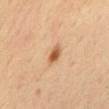A close-up tile cropped from a whole-body skin photograph, about 15 mm across. The tile uses cross-polarized illumination. Automated tile analysis of the lesion measured a lesion area of about 3.5 mm² and a symmetry-axis asymmetry near 0.3. And it measured border irregularity of about 2.5 on a 0–10 scale, a within-lesion color-variation index near 4.5/10, and a peripheral color-asymmetry measure near 1.5. And it measured a classifier nevus-likeness of about 95/100 and lesion-presence confidence of about 100/100. Located on the mid back. Measured at roughly 2.5 mm in maximum diameter. A male patient aged approximately 60.